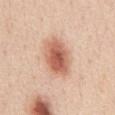This lesion was catalogued during total-body skin photography and was not selected for biopsy.
A roughly 15 mm field-of-view crop from a total-body skin photograph.
This is a white-light tile.
A female patient, about 45 years old.
Approximately 5 mm at its widest.
On the chest.
Automated tile analysis of the lesion measured a border-irregularity index near 1.5/10 and radial color variation of about 1.5. The analysis additionally found a nevus-likeness score of about 100/100 and a lesion-detection confidence of about 100/100.